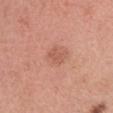The lesion was photographed on a routine skin check and not biopsied; there is no pathology result. A roughly 15 mm field-of-view crop from a total-body skin photograph. A female subject aged 18–22. The lesion is on the head or neck. The tile uses white-light illumination. Approximately 2.5 mm at its widest. Automated tile analysis of the lesion measured a lesion–skin lightness drop of about 7 and a normalized border contrast of about 5.5. It also reported a nevus-likeness score of about 5/100 and a detector confidence of about 100 out of 100 that the crop contains a lesion.